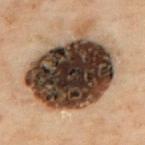– follow-up · imaged on a skin check; not biopsied
– subject · male, aged approximately 50
– site · the upper back
– lesion size · about 9.5 mm
– lighting · cross-polarized
– image · ~15 mm crop, total-body skin-cancer survey
– automated lesion analysis · a lesion area of about 60 mm², an eccentricity of roughly 0.6, and two-axis asymmetry of about 0.2; a lesion color around L≈29 a*≈11 b*≈20 in CIELAB and a lesion-to-skin contrast of about 20.5 (normalized; higher = more distinct); a border-irregularity index near 2.5/10, a color-variation rating of about 9.5/10, and a peripheral color-asymmetry measure near 3